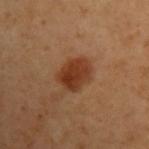On the arm.
The lesion's longest dimension is about 4 mm.
Imaged with cross-polarized lighting.
The patient is a male aged approximately 50.
A lesion tile, about 15 mm wide, cut from a 3D total-body photograph.